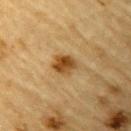Part of a total-body skin-imaging series; this lesion was reviewed on a skin check and was not flagged for biopsy.
The lesion's longest dimension is about 3 mm.
From the left upper arm.
The lesion-visualizer software estimated a lesion color around L≈41 a*≈17 b*≈37 in CIELAB, a lesion–skin lightness drop of about 12, and a normalized border contrast of about 10. And it measured a classifier nevus-likeness of about 95/100 and lesion-presence confidence of about 100/100.
A male subject, aged 83 or older.
This is a cross-polarized tile.
Cropped from a total-body skin-imaging series; the visible field is about 15 mm.